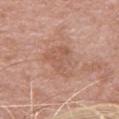No biopsy was performed on this lesion — it was imaged during a full skin examination and was not determined to be concerning. The lesion is on the upper back. The total-body-photography lesion software estimated a lesion color around L≈57 a*≈22 b*≈29 in CIELAB, a lesion–skin lightness drop of about 7, and a normalized lesion–skin contrast near 5. It also reported a border-irregularity rating of about 5.5/10 and peripheral color asymmetry of about 0.5. And it measured a nevus-likeness score of about 0/100 and lesion-presence confidence of about 100/100. A 15 mm crop from a total-body photograph taken for skin-cancer surveillance. The tile uses white-light illumination. The recorded lesion diameter is about 4.5 mm. A female patient, approximately 65 years of age.